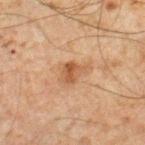<case>
  <biopsy_status>not biopsied; imaged during a skin examination</biopsy_status>
  <image>
    <source>total-body photography crop</source>
    <field_of_view_mm>15</field_of_view_mm>
  </image>
  <patient>
    <sex>male</sex>
    <age_approx>45</age_approx>
  </patient>
  <site>right lower leg</site>
  <lesion_size>
    <long_diameter_mm_approx>4.0</long_diameter_mm_approx>
  </lesion_size>
  <lighting>cross-polarized</lighting>
</case>